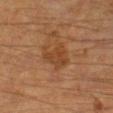biopsy_status: not biopsied; imaged during a skin examination
patient:
  sex: male
  age_approx: 65
site: right lower leg
image:
  source: total-body photography crop
  field_of_view_mm: 15